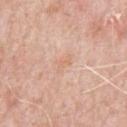– biopsy status — catalogued during a skin exam; not biopsied
– body site — the chest
– lighting — white-light
– lesion diameter — about 2.5 mm
– subject — male, in their 80s
– image — 15 mm crop, total-body photography